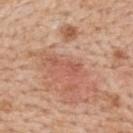Part of a total-body skin-imaging series; this lesion was reviewed on a skin check and was not flagged for biopsy.
On the upper back.
The patient is a female aged around 50.
A lesion tile, about 15 mm wide, cut from a 3D total-body photograph.
Measured at roughly 4 mm in maximum diameter.
The total-body-photography lesion software estimated a lesion area of about 4.5 mm² and a shape eccentricity near 0.95. It also reported an average lesion color of about L≈56 a*≈26 b*≈30 (CIELAB), a lesion–skin lightness drop of about 7, and a normalized lesion–skin contrast near 5. It also reported lesion-presence confidence of about 90/100.
The tile uses white-light illumination.